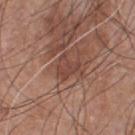Impression: This lesion was catalogued during total-body skin photography and was not selected for biopsy. Image and clinical context: Measured at roughly 2.5 mm in maximum diameter. The lesion is on the chest. A close-up tile cropped from a whole-body skin photograph, about 15 mm across. The patient is a male aged approximately 55.